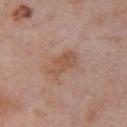image: ~15 mm tile from a whole-body skin photo
lesion size: ~4 mm (longest diameter)
subject: male, aged approximately 55
site: the arm